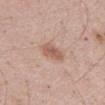Assessment: Part of a total-body skin-imaging series; this lesion was reviewed on a skin check and was not flagged for biopsy. Context: This is a white-light tile. A 15 mm crop from a total-body photograph taken for skin-cancer surveillance. Longest diameter approximately 3 mm. Located on the front of the torso. A male subject aged around 60.